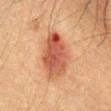follow-up — imaged on a skin check; not biopsied
image source — 15 mm crop, total-body photography
subject — male, aged 48 to 52
site — the front of the torso